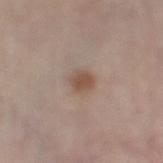Impression: Part of a total-body skin-imaging series; this lesion was reviewed on a skin check and was not flagged for biopsy.